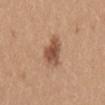Image and clinical context: From the mid back. A 15 mm close-up extracted from a 3D total-body photography capture. A female patient aged around 35. The tile uses white-light illumination. Longest diameter approximately 4 mm.